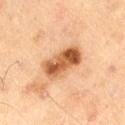No biopsy was performed on this lesion — it was imaged during a full skin examination and was not determined to be concerning. A 15 mm crop from a total-body photograph taken for skin-cancer surveillance. The subject is a male in their 70s. From the right thigh. Automated tile analysis of the lesion measured an average lesion color of about L≈47 a*≈21 b*≈34 (CIELAB), about 14 CIELAB-L* units darker than the surrounding skin, and a lesion-to-skin contrast of about 10.5 (normalized; higher = more distinct). This is a cross-polarized tile.